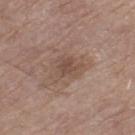Imaged during a routine full-body skin examination; the lesion was not biopsied and no histopathology is available.
Captured under white-light illumination.
The lesion is on the left thigh.
A male patient about 65 years old.
A 15 mm close-up extracted from a 3D total-body photography capture.